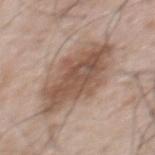Imaged during a routine full-body skin examination; the lesion was not biopsied and no histopathology is available. The total-body-photography lesion software estimated a lesion color around L≈53 a*≈17 b*≈27 in CIELAB, about 12 CIELAB-L* units darker than the surrounding skin, and a lesion-to-skin contrast of about 8.5 (normalized; higher = more distinct). The software also gave an automated nevus-likeness rating near 25 out of 100 and lesion-presence confidence of about 100/100. Imaged with white-light lighting. Cropped from a whole-body photographic skin survey; the tile spans about 15 mm. About 8 mm across. The subject is a male roughly 55 years of age. The lesion is on the upper back.